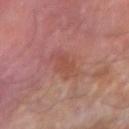{"image": {"source": "total-body photography crop", "field_of_view_mm": 15}, "patient": {"sex": "male", "age_approx": 65}, "site": "right forearm"}